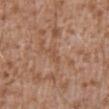biopsy status: no biopsy performed (imaged during a skin exam)
automated lesion analysis: a lesion area of about 2 mm², an outline eccentricity of about 0.95 (0 = round, 1 = elongated), and a symmetry-axis asymmetry near 0.3; a lesion color around L≈51 a*≈21 b*≈32 in CIELAB, roughly 6 lightness units darker than nearby skin, and a normalized lesion–skin contrast near 4.5; border irregularity of about 4 on a 0–10 scale, a within-lesion color-variation index near 0/10, and a peripheral color-asymmetry measure near 0
lesion diameter: ≈2.5 mm
patient: male, in their mid-40s
anatomic site: the front of the torso
tile lighting: white-light
acquisition: ~15 mm crop, total-body skin-cancer survey